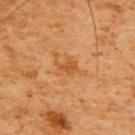Clinical impression:
Imaged during a routine full-body skin examination; the lesion was not biopsied and no histopathology is available.
Image and clinical context:
The lesion is located on the upper back. A male subject, aged around 60. Cropped from a total-body skin-imaging series; the visible field is about 15 mm. An algorithmic analysis of the crop reported a shape eccentricity near 0.7. It also reported a border-irregularity rating of about 4.5/10, a color-variation rating of about 1.5/10, and peripheral color asymmetry of about 0.5. Captured under cross-polarized illumination.